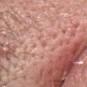Image and clinical context: An algorithmic analysis of the crop reported an area of roughly 1 mm², an outline eccentricity of about 0.85 (0 = round, 1 = elongated), and two-axis asymmetry of about 0.35. And it measured a border-irregularity index near 3/10 and internal color variation of about 0 on a 0–10 scale. The software also gave lesion-presence confidence of about 65/100. The lesion is located on the head or neck. This is a white-light tile. This image is a 15 mm lesion crop taken from a total-body photograph. Measured at roughly 1.5 mm in maximum diameter. The subject is a female in their mid-50s. Conclusion: The biopsy diagnosis was an intradermal melanocytic nevus — a benign skin lesion.